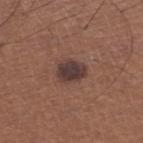<record>
<image>
  <source>total-body photography crop</source>
  <field_of_view_mm>15</field_of_view_mm>
</image>
<patient>
  <sex>male</sex>
  <age_approx>65</age_approx>
</patient>
<site>right lower leg</site>
</record>